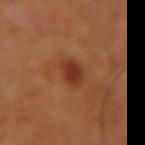notes = imaged on a skin check; not biopsied | subject = male, aged 48–52 | lesion diameter = ≈3 mm | image source = total-body-photography crop, ~15 mm field of view | anatomic site = the right upper arm | tile lighting = cross-polarized illumination.